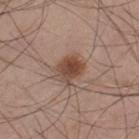Recorded during total-body skin imaging; not selected for excision or biopsy. Measured at roughly 4 mm in maximum diameter. The tile uses white-light illumination. From the left thigh. The lesion-visualizer software estimated a shape-asymmetry score of about 0.2 (0 = symmetric). It also reported a border-irregularity rating of about 2.5/10 and peripheral color asymmetry of about 2. A male subject roughly 45 years of age. A 15 mm crop from a total-body photograph taken for skin-cancer surveillance.